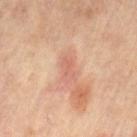{"biopsy_status": "not biopsied; imaged during a skin examination", "site": "right leg", "image": {"source": "total-body photography crop", "field_of_view_mm": 15}, "patient": {"sex": "female", "age_approx": 65}, "automated_metrics": {"area_mm2_approx": 3.5, "eccentricity": 0.85, "shape_asymmetry": 0.35, "border_irregularity_0_10": 3.0, "color_variation_0_10": 2.5, "peripheral_color_asymmetry": 1.0}}